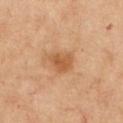Part of a total-body skin-imaging series; this lesion was reviewed on a skin check and was not flagged for biopsy. The tile uses cross-polarized illumination. On the chest. A lesion tile, about 15 mm wide, cut from a 3D total-body photograph. A female subject, aged 43 to 47. Measured at roughly 3 mm in maximum diameter.